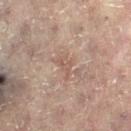<case>
  <biopsy_status>not biopsied; imaged during a skin examination</biopsy_status>
  <site>left leg</site>
  <lesion_size>
    <long_diameter_mm_approx>3.0</long_diameter_mm_approx>
  </lesion_size>
  <image>
    <source>total-body photography crop</source>
    <field_of_view_mm>15</field_of_view_mm>
  </image>
  <patient>
    <sex>female</sex>
    <age_approx>80</age_approx>
  </patient>
  <automated_metrics>
    <area_mm2_approx>3.0</area_mm2_approx>
    <eccentricity>0.85</eccentricity>
    <shape_asymmetry>0.55</shape_asymmetry>
    <cielab_L>50</cielab_L>
    <cielab_a>17</cielab_a>
    <cielab_b>23</cielab_b>
    <vs_skin_darker_L>6.0</vs_skin_darker_L>
    <nevus_likeness_0_100>0</nevus_likeness_0_100>
    <lesion_detection_confidence_0_100>70</lesion_detection_confidence_0_100>
  </automated_metrics>
</case>